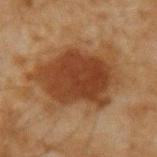  biopsy_status: not biopsied; imaged during a skin examination
  site: left upper arm
  lighting: cross-polarized
  patient:
    sex: male
    age_approx: 45
  image:
    source: total-body photography crop
    field_of_view_mm: 15
  lesion_size:
    long_diameter_mm_approx: 9.0